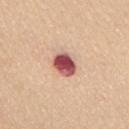notes: no biopsy performed (imaged during a skin exam) | location: the mid back | image: ~15 mm crop, total-body skin-cancer survey | patient: female, aged 63 to 67 | tile lighting: white-light | lesion size: ~3.5 mm (longest diameter) | automated metrics: a border-irregularity index near 1.5/10; a nevus-likeness score of about 0/100 and a detector confidence of about 100 out of 100 that the crop contains a lesion.